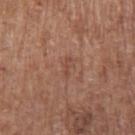  biopsy_status: not biopsied; imaged during a skin examination
  lesion_size:
    long_diameter_mm_approx: 2.5
  automated_metrics:
    area_mm2_approx: 2.5
    eccentricity: 0.9
    shape_asymmetry: 0.35
    border_irregularity_0_10: 4.5
    color_variation_0_10: 0.0
    peripheral_color_asymmetry: 0.0
  image:
    source: total-body photography crop
    field_of_view_mm: 15
  site: right upper arm
  lighting: white-light
  patient:
    sex: male
    age_approx: 65